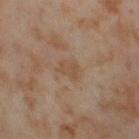Recorded during total-body skin imaging; not selected for excision or biopsy. The subject is a female approximately 55 years of age. This is a cross-polarized tile. Automated image analysis of the tile measured a shape-asymmetry score of about 0.35 (0 = symmetric). A close-up tile cropped from a whole-body skin photograph, about 15 mm across. The recorded lesion diameter is about 3 mm. Located on the right thigh.